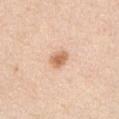The lesion was tiled from a total-body skin photograph and was not biopsied. The recorded lesion diameter is about 2.5 mm. Imaged with white-light lighting. The patient is a male aged approximately 50. On the left upper arm. A 15 mm crop from a total-body photograph taken for skin-cancer surveillance.